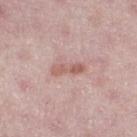The lesion was tiled from a total-body skin photograph and was not biopsied.
An algorithmic analysis of the crop reported a footprint of about 5 mm², a shape eccentricity near 0.95, and a symmetry-axis asymmetry near 0.25. The analysis additionally found a border-irregularity index near 3/10, a color-variation rating of about 4/10, and peripheral color asymmetry of about 1.5.
The subject is a female roughly 45 years of age.
On the right thigh.
About 4 mm across.
Imaged with white-light lighting.
A 15 mm close-up tile from a total-body photography series done for melanoma screening.